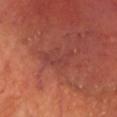The lesion was photographed on a routine skin check and not biopsied; there is no pathology result. A male patient aged 63 to 67. A close-up tile cropped from a whole-body skin photograph, about 15 mm across. On the head or neck.